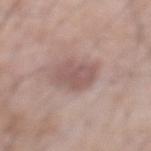The lesion was photographed on a routine skin check and not biopsied; there is no pathology result. Cropped from a whole-body photographic skin survey; the tile spans about 15 mm. The lesion's longest dimension is about 3.5 mm. A male subject approximately 70 years of age. This is a white-light tile. Located on the abdomen. The lesion-visualizer software estimated an outline eccentricity of about 0.6 (0 = round, 1 = elongated) and a symmetry-axis asymmetry near 0.15. The software also gave a lesion color around L≈54 a*≈19 b*≈21 in CIELAB, about 9 CIELAB-L* units darker than the surrounding skin, and a normalized lesion–skin contrast near 6. The software also gave a color-variation rating of about 2/10 and peripheral color asymmetry of about 0.5. And it measured a classifier nevus-likeness of about 10/100 and lesion-presence confidence of about 100/100.